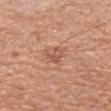Findings:
- follow-up: no biopsy performed (imaged during a skin exam)
- subject: female, in their 50s
- location: the arm
- diameter: about 2.5 mm
- image: total-body-photography crop, ~15 mm field of view